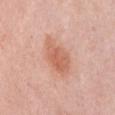No biopsy was performed on this lesion — it was imaged during a full skin examination and was not determined to be concerning.
The lesion's longest dimension is about 5 mm.
A roughly 15 mm field-of-view crop from a total-body skin photograph.
A female patient, roughly 35 years of age.
Located on the front of the torso.
The lesion-visualizer software estimated a mean CIELAB color near L≈62 a*≈25 b*≈32, roughly 10 lightness units darker than nearby skin, and a normalized lesion–skin contrast near 7.
Captured under white-light illumination.